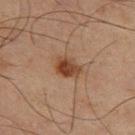workup = imaged on a skin check; not biopsied
subject = male, roughly 65 years of age
lesion diameter = ~3.5 mm (longest diameter)
anatomic site = the left thigh
tile lighting = cross-polarized
acquisition = ~15 mm crop, total-body skin-cancer survey
automated lesion analysis = a border-irregularity rating of about 3/10, a color-variation rating of about 4/10, and peripheral color asymmetry of about 1.5; a nevus-likeness score of about 90/100 and lesion-presence confidence of about 100/100